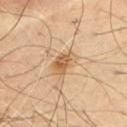<lesion>
<biopsy_status>not biopsied; imaged during a skin examination</biopsy_status>
<image>
  <source>total-body photography crop</source>
  <field_of_view_mm>15</field_of_view_mm>
</image>
<patient>
  <sex>male</sex>
  <age_approx>55</age_approx>
</patient>
<site>chest</site>
<lighting>cross-polarized</lighting>
</lesion>